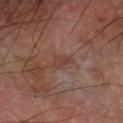Impression: The lesion was photographed on a routine skin check and not biopsied; there is no pathology result. Acquisition and patient details: A male subject, aged 68–72. The lesion is on the arm. A 15 mm close-up tile from a total-body photography series done for melanoma screening. Longest diameter approximately 3 mm.